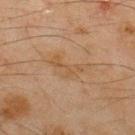{"biopsy_status": "not biopsied; imaged during a skin examination", "patient": {"sex": "male", "age_approx": 45}, "image": {"source": "total-body photography crop", "field_of_view_mm": 15}, "site": "upper back"}